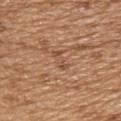{
  "biopsy_status": "not biopsied; imaged during a skin examination",
  "patient": {
    "sex": "male",
    "age_approx": 70
  },
  "lesion_size": {
    "long_diameter_mm_approx": 2.5
  },
  "image": {
    "source": "total-body photography crop",
    "field_of_view_mm": 15
  },
  "site": "head or neck",
  "lighting": "white-light"
}